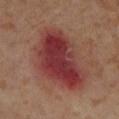Findings:
• follow-up · catalogued during a skin exam; not biopsied
• body site · the right lower leg
• illumination · cross-polarized illumination
• patient · female, roughly 55 years of age
• lesion size · ~8.5 mm (longest diameter)
• image · total-body-photography crop, ~15 mm field of view